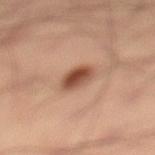Imaged during a routine full-body skin examination; the lesion was not biopsied and no histopathology is available. A lesion tile, about 15 mm wide, cut from a 3D total-body photograph. From the left lower leg. The subject is a male about 40 years old.